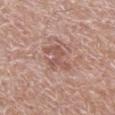Impression:
The lesion was photographed on a routine skin check and not biopsied; there is no pathology result.